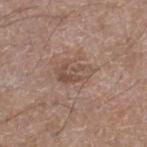Findings:
- notes: total-body-photography surveillance lesion; no biopsy
- location: the right lower leg
- lesion size: ~4 mm (longest diameter)
- illumination: white-light illumination
- acquisition: 15 mm crop, total-body photography
- image-analysis metrics: a lesion area of about 7.5 mm², an eccentricity of roughly 0.85, and a shape-asymmetry score of about 0.3 (0 = symmetric); a mean CIELAB color near L≈50 a*≈17 b*≈24, a lesion–skin lightness drop of about 8, and a normalized lesion–skin contrast near 6
- subject: male, approximately 60 years of age